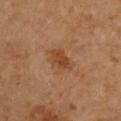Case summary:
* acquisition: ~15 mm tile from a whole-body skin photo
* illumination: cross-polarized
* patient: male, in their mid-60s
* automated metrics: a shape-asymmetry score of about 0.3 (0 = symmetric); about 7 CIELAB-L* units darker than the surrounding skin and a normalized lesion–skin contrast near 6.5; a border-irregularity index near 3/10
* lesion size: ~4 mm (longest diameter)
* body site: the left arm